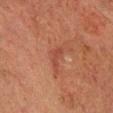Cropped from a whole-body photographic skin survey; the tile spans about 15 mm. Captured under cross-polarized illumination. On the head or neck. A male patient in their mid-60s.